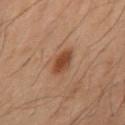biopsy status = total-body-photography surveillance lesion; no biopsy | location = the mid back | image source = 15 mm crop, total-body photography | lighting = cross-polarized | subject = male, aged approximately 50.